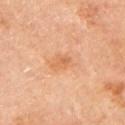Imaged during a routine full-body skin examination; the lesion was not biopsied and no histopathology is available.
The tile uses cross-polarized illumination.
Cropped from a total-body skin-imaging series; the visible field is about 15 mm.
A female patient, roughly 65 years of age.
From the left upper arm.
The lesion's longest dimension is about 2.5 mm.